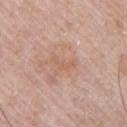Clinical impression:
Part of a total-body skin-imaging series; this lesion was reviewed on a skin check and was not flagged for biopsy.
Acquisition and patient details:
The subject is a male about 65 years old. The lesion is on the left upper arm. Captured under white-light illumination. Cropped from a total-body skin-imaging series; the visible field is about 15 mm. The recorded lesion diameter is about 3 mm.